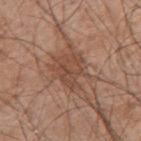Assessment: Captured during whole-body skin photography for melanoma surveillance; the lesion was not biopsied. Image and clinical context: A male subject about 65 years old. Measured at roughly 7 mm in maximum diameter. From the arm. Cropped from a total-body skin-imaging series; the visible field is about 15 mm. The tile uses white-light illumination.